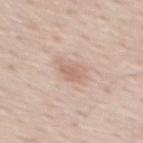biopsy status=no biopsy performed (imaged during a skin exam) | lighting=white-light illumination | patient=male, aged approximately 55 | imaging modality=~15 mm tile from a whole-body skin photo | site=the mid back.